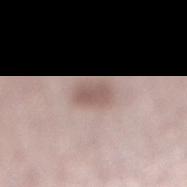Clinical impression: Part of a total-body skin-imaging series; this lesion was reviewed on a skin check and was not flagged for biopsy. Clinical summary: A region of skin cropped from a whole-body photographic capture, roughly 15 mm wide. The lesion is located on the right lower leg. The patient is a female in their mid-50s.